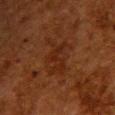No biopsy was performed on this lesion — it was imaged during a full skin examination and was not determined to be concerning.
On the upper back.
A female subject, aged 48 to 52.
A 15 mm close-up tile from a total-body photography series done for melanoma screening.